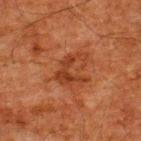notes: catalogued during a skin exam; not biopsied | imaging modality: total-body-photography crop, ~15 mm field of view | lesion diameter: ~4 mm (longest diameter) | patient: male, approximately 60 years of age | automated lesion analysis: a lesion area of about 7.5 mm², a shape eccentricity near 0.6, and a shape-asymmetry score of about 0.65 (0 = symmetric); a mean CIELAB color near L≈31 a*≈24 b*≈30, about 7 CIELAB-L* units darker than the surrounding skin, and a normalized border contrast of about 6.5 | illumination: cross-polarized | body site: the upper back.